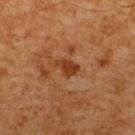Assessment: Part of a total-body skin-imaging series; this lesion was reviewed on a skin check and was not flagged for biopsy. Clinical summary: An algorithmic analysis of the crop reported a border-irregularity rating of about 3/10, a within-lesion color-variation index near 1/10, and a peripheral color-asymmetry measure near 0.5. Measured at roughly 2.5 mm in maximum diameter. A male subject, aged 58–62. Imaged with cross-polarized lighting. This image is a 15 mm lesion crop taken from a total-body photograph. On the upper back.